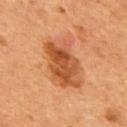notes=total-body-photography surveillance lesion; no biopsy
site=the mid back
image source=total-body-photography crop, ~15 mm field of view
image-analysis metrics=lesion-presence confidence of about 100/100
illumination=cross-polarized illumination
subject=male, roughly 55 years of age